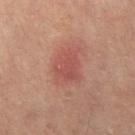Q: Was a biopsy performed?
A: catalogued during a skin exam; not biopsied